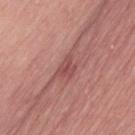Imaged during a routine full-body skin examination; the lesion was not biopsied and no histopathology is available. A female subject aged 63–67. A 15 mm close-up extracted from a 3D total-body photography capture. About 2.5 mm across. Captured under white-light illumination. Automated image analysis of the tile measured an area of roughly 3.5 mm² and a shape eccentricity near 0.6. The analysis additionally found a lesion color around L≈49 a*≈26 b*≈23 in CIELAB, a lesion–skin lightness drop of about 9, and a normalized lesion–skin contrast near 6. The lesion is located on the left thigh.